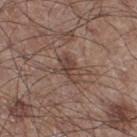The total-body-photography lesion software estimated a footprint of about 6 mm², an eccentricity of roughly 0.75, and two-axis asymmetry of about 0.35. It also reported a border-irregularity rating of about 5/10. It also reported a classifier nevus-likeness of about 0/100 and lesion-presence confidence of about 100/100.
Approximately 3.5 mm at its widest.
A male patient aged approximately 60.
Cropped from a whole-body photographic skin survey; the tile spans about 15 mm.
Captured under white-light illumination.
Located on the right thigh.